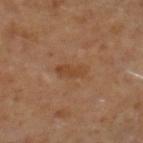biopsy_status: not biopsied; imaged during a skin examination
image:
  source: total-body photography crop
  field_of_view_mm: 15
lighting: cross-polarized
automated_metrics:
  nevus_likeness_0_100: 5
site: leg
lesion_size:
  long_diameter_mm_approx: 3.5
patient:
  sex: male
  age_approx: 60